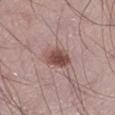Background: Located on the left lower leg. A male patient roughly 50 years of age. A close-up tile cropped from a whole-body skin photograph, about 15 mm across.